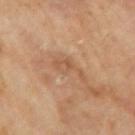Part of a total-body skin-imaging series; this lesion was reviewed on a skin check and was not flagged for biopsy.
The subject is a male aged 63–67.
Automated tile analysis of the lesion measured a mean CIELAB color near L≈52 a*≈19 b*≈32, a lesion–skin lightness drop of about 7, and a lesion-to-skin contrast of about 5 (normalized; higher = more distinct). And it measured a border-irregularity index near 6.5/10, a color-variation rating of about 3/10, and peripheral color asymmetry of about 1.
A close-up tile cropped from a whole-body skin photograph, about 15 mm across.
The lesion is located on the right upper arm.